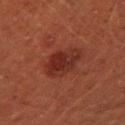| feature | finding |
|---|---|
| site | the right upper arm |
| illumination | cross-polarized illumination |
| patient | male, in their mid- to late 40s |
| diameter | ~4 mm (longest diameter) |
| image | ~15 mm crop, total-body skin-cancer survey |